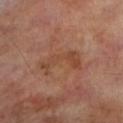Clinical summary: A male subject, about 70 years old. About 5 mm across. The total-body-photography lesion software estimated a border-irregularity rating of about 7.5/10 and radial color variation of about 1.5. And it measured a detector confidence of about 100 out of 100 that the crop contains a lesion. The lesion is on the leg. Imaged with cross-polarized lighting. A roughly 15 mm field-of-view crop from a total-body skin photograph.